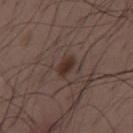Recorded during total-body skin imaging; not selected for excision or biopsy. Cropped from a whole-body photographic skin survey; the tile spans about 15 mm. Imaged with white-light lighting. Approximately 2.5 mm at its widest. The lesion is on the back. The subject is a male approximately 30 years of age. The total-body-photography lesion software estimated an eccentricity of roughly 0.8 and a symmetry-axis asymmetry near 0.2. The analysis additionally found a mean CIELAB color near L≈31 a*≈16 b*≈21, roughly 9 lightness units darker than nearby skin, and a lesion-to-skin contrast of about 9 (normalized; higher = more distinct). And it measured radial color variation of about 1. The analysis additionally found a classifier nevus-likeness of about 95/100 and a detector confidence of about 100 out of 100 that the crop contains a lesion.